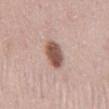Clinical impression: Part of a total-body skin-imaging series; this lesion was reviewed on a skin check and was not flagged for biopsy. Context: On the abdomen. A 15 mm crop from a total-body photograph taken for skin-cancer surveillance. Captured under white-light illumination. A female subject roughly 30 years of age.